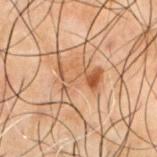biopsy_status: not biopsied; imaged during a skin examination
patient:
  sex: male
  age_approx: 50
lighting: cross-polarized
site: chest
lesion_size:
  long_diameter_mm_approx: 4.5
image:
  source: total-body photography crop
  field_of_view_mm: 15